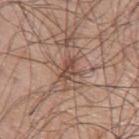The subject is a male aged approximately 65. Imaged with white-light lighting. A close-up tile cropped from a whole-body skin photograph, about 15 mm across. Measured at roughly 3 mm in maximum diameter. On the left upper arm. The lesion-visualizer software estimated a mean CIELAB color near L≈48 a*≈19 b*≈26 and roughly 10 lightness units darker than nearby skin. The analysis additionally found a border-irregularity index near 6/10, a within-lesion color-variation index near 5/10, and peripheral color asymmetry of about 2.